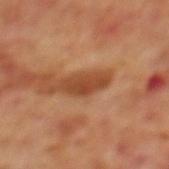workup — catalogued during a skin exam; not biopsied
patient — male, approximately 70 years of age
anatomic site — the back
automated metrics — an area of roughly 6 mm², an outline eccentricity of about 0.9 (0 = round, 1 = elongated), and a shape-asymmetry score of about 0.25 (0 = symmetric); a mean CIELAB color near L≈43 a*≈25 b*≈35, a lesion–skin lightness drop of about 11, and a normalized border contrast of about 8.5
illumination — cross-polarized
acquisition — 15 mm crop, total-body photography